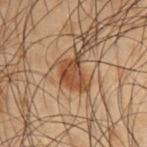The lesion's longest dimension is about 4 mm. A male subject aged 48 to 52. The lesion-visualizer software estimated an eccentricity of roughly 0.75. The software also gave an average lesion color of about L≈35 a*≈17 b*≈27 (CIELAB) and roughly 10 lightness units darker than nearby skin. The software also gave a border-irregularity index near 5/10, a within-lesion color-variation index near 3/10, and peripheral color asymmetry of about 1. Imaged with cross-polarized lighting. A lesion tile, about 15 mm wide, cut from a 3D total-body photograph. From the right upper arm.